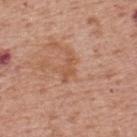{"biopsy_status": "not biopsied; imaged during a skin examination", "lesion_size": {"long_diameter_mm_approx": 3.5}, "lighting": "white-light", "image": {"source": "total-body photography crop", "field_of_view_mm": 15}, "patient": {"sex": "male", "age_approx": 70}, "site": "upper back"}